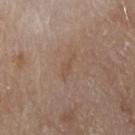Recorded during total-body skin imaging; not selected for excision or biopsy. About 3 mm across. The lesion is on the front of the torso. The tile uses white-light illumination. A 15 mm close-up tile from a total-body photography series done for melanoma screening. A male subject, about 80 years old.